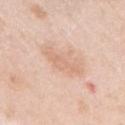Q: Is there a histopathology result?
A: catalogued during a skin exam; not biopsied
Q: Lesion location?
A: the right upper arm
Q: What lighting was used for the tile?
A: white-light
Q: What is the lesion's diameter?
A: ~4 mm (longest diameter)
Q: How was this image acquired?
A: total-body-photography crop, ~15 mm field of view
Q: Who is the patient?
A: female, about 65 years old
Q: Automated lesion metrics?
A: a footprint of about 5 mm², a shape eccentricity near 0.9, and a symmetry-axis asymmetry near 0.3; a mean CIELAB color near L≈69 a*≈20 b*≈31 and about 7 CIELAB-L* units darker than the surrounding skin; an automated nevus-likeness rating near 0 out of 100 and lesion-presence confidence of about 100/100